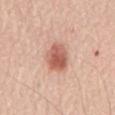Q: Was a biopsy performed?
A: imaged on a skin check; not biopsied
Q: How was the tile lit?
A: white-light
Q: What is the lesion's diameter?
A: ≈3.5 mm
Q: How was this image acquired?
A: 15 mm crop, total-body photography
Q: Lesion location?
A: the mid back
Q: Who is the patient?
A: male, about 70 years old